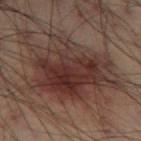biopsy_status: not biopsied; imaged during a skin examination
image:
  source: total-body photography crop
  field_of_view_mm: 15
lighting: cross-polarized
patient:
  sex: male
  age_approx: 55
lesion_size:
  long_diameter_mm_approx: 10.0
site: leg